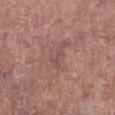Assessment: Imaged during a routine full-body skin examination; the lesion was not biopsied and no histopathology is available. Acquisition and patient details: On the left lower leg. Automated image analysis of the tile measured a lesion area of about 3 mm², a shape eccentricity near 0.8, and a symmetry-axis asymmetry near 0.5. It also reported about 6 CIELAB-L* units darker than the surrounding skin and a lesion-to-skin contrast of about 4.5 (normalized; higher = more distinct). The software also gave a nevus-likeness score of about 0/100 and a lesion-detection confidence of about 95/100. Imaged with white-light lighting. A roughly 15 mm field-of-view crop from a total-body skin photograph. Approximately 3 mm at its widest. A male subject, aged 63–67.